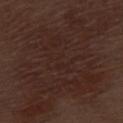biopsy status = imaged on a skin check; not biopsied
subject = male, approximately 70 years of age
lesion size = ≈17.5 mm
body site = the left thigh
image = total-body-photography crop, ~15 mm field of view
automated metrics = a border-irregularity rating of about 7.5/10, a color-variation rating of about 4/10, and radial color variation of about 1.5